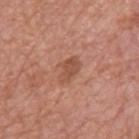Located on the mid back.
About 3.5 mm across.
Cropped from a total-body skin-imaging series; the visible field is about 15 mm.
This is a white-light tile.
A male subject, approximately 65 years of age.
Automated tile analysis of the lesion measured a shape-asymmetry score of about 0.25 (0 = symmetric). It also reported a classifier nevus-likeness of about 5/100 and a lesion-detection confidence of about 100/100.